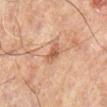{
  "biopsy_status": "not biopsied; imaged during a skin examination",
  "patient": {
    "sex": "male",
    "age_approx": 60
  },
  "site": "leg",
  "image": {
    "source": "total-body photography crop",
    "field_of_view_mm": 15
  },
  "lighting": "cross-polarized",
  "lesion_size": {
    "long_diameter_mm_approx": 2.5
  }
}